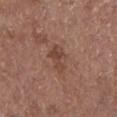Impression:
The lesion was photographed on a routine skin check and not biopsied; there is no pathology result.
Clinical summary:
Approximately 3.5 mm at its widest. The total-body-photography lesion software estimated an area of roughly 6 mm² and a shape-asymmetry score of about 0.3 (0 = symmetric). The analysis additionally found a classifier nevus-likeness of about 0/100 and a lesion-detection confidence of about 100/100. On the left lower leg. Cropped from a total-body skin-imaging series; the visible field is about 15 mm. The patient is a male about 75 years old. Captured under white-light illumination.